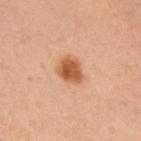Case summary:
• workup · no biopsy performed (imaged during a skin exam)
• subject · male, about 60 years old
• diameter · about 3.5 mm
• body site · the right upper arm
• automated lesion analysis · a footprint of about 6.5 mm² and an outline eccentricity of about 0.65 (0 = round, 1 = elongated); a lesion color around L≈42 a*≈20 b*≈30 in CIELAB and a lesion–skin lightness drop of about 11
• image · total-body-photography crop, ~15 mm field of view